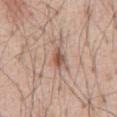The lesion was tiled from a total-body skin photograph and was not biopsied. A male patient in their mid-50s. From the abdomen. This image is a 15 mm lesion crop taken from a total-body photograph. Measured at roughly 2.5 mm in maximum diameter. The lesion-visualizer software estimated a border-irregularity rating of about 3/10, a within-lesion color-variation index near 5.5/10, and radial color variation of about 2.